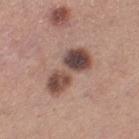The lesion was photographed on a routine skin check and not biopsied; there is no pathology result.
The total-body-photography lesion software estimated a footprint of about 14 mm², an eccentricity of roughly 0.9, and a shape-asymmetry score of about 0.35 (0 = symmetric). The analysis additionally found a lesion color around L≈46 a*≈18 b*≈23 in CIELAB, about 16 CIELAB-L* units darker than the surrounding skin, and a normalized lesion–skin contrast near 11. It also reported border irregularity of about 5 on a 0–10 scale, a within-lesion color-variation index near 10/10, and a peripheral color-asymmetry measure near 3.
Located on the leg.
A 15 mm crop from a total-body photograph taken for skin-cancer surveillance.
Approximately 6 mm at its widest.
A female subject aged approximately 30.
Imaged with white-light lighting.